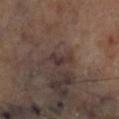biopsy status = catalogued during a skin exam; not biopsied | anatomic site = the leg | lesion diameter = ~3 mm (longest diameter) | lighting = cross-polarized | acquisition = total-body-photography crop, ~15 mm field of view.